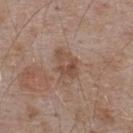No biopsy was performed on this lesion — it was imaged during a full skin examination and was not determined to be concerning. A male subject, aged approximately 55. Longest diameter approximately 4 mm. The lesion is on the back. The tile uses white-light illumination. A region of skin cropped from a whole-body photographic capture, roughly 15 mm wide. The total-body-photography lesion software estimated an area of roughly 8.5 mm², a shape eccentricity near 0.75, and a symmetry-axis asymmetry near 0.35. It also reported a mean CIELAB color near L≈49 a*≈18 b*≈27, a lesion–skin lightness drop of about 8, and a normalized lesion–skin contrast near 6. The software also gave a border-irregularity index near 3.5/10, internal color variation of about 5 on a 0–10 scale, and peripheral color asymmetry of about 2. And it measured a detector confidence of about 100 out of 100 that the crop contains a lesion.